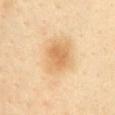No biopsy was performed on this lesion — it was imaged during a full skin examination and was not determined to be concerning.
Cropped from a whole-body photographic skin survey; the tile spans about 15 mm.
Captured under cross-polarized illumination.
The patient is a male aged around 40.
Measured at roughly 6 mm in maximum diameter.
Automated image analysis of the tile measured a border-irregularity index near 2.5/10, a color-variation rating of about 5.5/10, and a peripheral color-asymmetry measure near 1.
From the left upper arm.